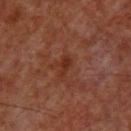Background: A male subject, aged around 60. The lesion's longest dimension is about 2.5 mm. Automated tile analysis of the lesion measured an average lesion color of about L≈28 a*≈23 b*≈28 (CIELAB), a lesion–skin lightness drop of about 6, and a lesion-to-skin contrast of about 7 (normalized; higher = more distinct). The software also gave a peripheral color-asymmetry measure near 0. And it measured a nevus-likeness score of about 0/100 and lesion-presence confidence of about 100/100. Imaged with cross-polarized lighting. The lesion is located on the head or neck. A 15 mm crop from a total-body photograph taken for skin-cancer surveillance.